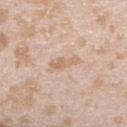Clinical impression: Recorded during total-body skin imaging; not selected for excision or biopsy. Context: A female subject aged 23–27. The lesion is located on the upper back. Imaged with white-light lighting. Longest diameter approximately 3.5 mm. Automated tile analysis of the lesion measured a footprint of about 5 mm² and an eccentricity of roughly 0.9. And it measured an automated nevus-likeness rating near 0 out of 100. This image is a 15 mm lesion crop taken from a total-body photograph.